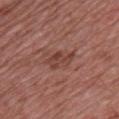{
  "lighting": "white-light",
  "automated_metrics": {
    "eccentricity": 0.85,
    "shape_asymmetry": 0.4,
    "cielab_L": 42,
    "cielab_a": 24,
    "cielab_b": 24,
    "vs_skin_darker_L": 8.0,
    "vs_skin_contrast_norm": 6.5,
    "border_irregularity_0_10": 5.0,
    "color_variation_0_10": 3.0,
    "peripheral_color_asymmetry": 1.0,
    "nevus_likeness_0_100": 0,
    "lesion_detection_confidence_0_100": 100
  },
  "patient": {
    "sex": "male",
    "age_approx": 50
  },
  "site": "chest",
  "lesion_size": {
    "long_diameter_mm_approx": 3.5
  },
  "image": {
    "source": "total-body photography crop",
    "field_of_view_mm": 15
  }
}